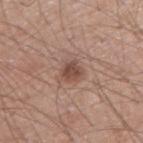This lesion was catalogued during total-body skin photography and was not selected for biopsy. Imaged with white-light lighting. An algorithmic analysis of the crop reported a mean CIELAB color near L≈49 a*≈19 b*≈25, about 10 CIELAB-L* units darker than the surrounding skin, and a lesion-to-skin contrast of about 7.5 (normalized; higher = more distinct). It also reported radial color variation of about 1. A 15 mm crop from a total-body photograph taken for skin-cancer surveillance. The recorded lesion diameter is about 3 mm. A male patient about 55 years old.